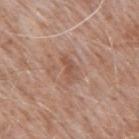The subject is a male roughly 50 years of age. Imaged with white-light lighting. On the upper back. A lesion tile, about 15 mm wide, cut from a 3D total-body photograph.